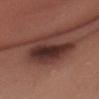Clinical impression: This lesion was catalogued during total-body skin photography and was not selected for biopsy. Acquisition and patient details: A 15 mm crop from a total-body photograph taken for skin-cancer surveillance. Captured under white-light illumination. A male subject, in their mid- to late 20s. The lesion is located on the head or neck. About 7 mm across.